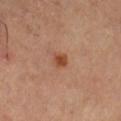Recorded during total-body skin imaging; not selected for excision or biopsy. A female patient in their 40s. Longest diameter approximately 2 mm. A 15 mm crop from a total-body photograph taken for skin-cancer surveillance. The lesion is on the left thigh.